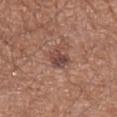The lesion was tiled from a total-body skin photograph and was not biopsied. A male patient, aged approximately 70. The total-body-photography lesion software estimated a mean CIELAB color near L≈45 a*≈21 b*≈23, about 10 CIELAB-L* units darker than the surrounding skin, and a lesion-to-skin contrast of about 8.5 (normalized; higher = more distinct). It also reported border irregularity of about 3.5 on a 0–10 scale and radial color variation of about 1.5. The tile uses white-light illumination. About 3 mm across. On the arm. Cropped from a whole-body photographic skin survey; the tile spans about 15 mm.